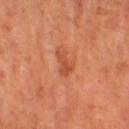{
  "biopsy_status": "not biopsied; imaged during a skin examination",
  "site": "right thigh",
  "lesion_size": {
    "long_diameter_mm_approx": 3.0
  },
  "patient": {
    "sex": "female",
    "age_approx": 65
  },
  "image": {
    "source": "total-body photography crop",
    "field_of_view_mm": 15
  },
  "lighting": "cross-polarized"
}